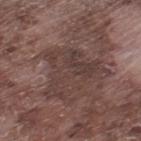biopsy_status: not biopsied; imaged during a skin examination
site: left thigh
automated_metrics:
  area_mm2_approx: 21.0
  eccentricity: 0.7
  shape_asymmetry: 0.7
  vs_skin_darker_L: 6.0
  vs_skin_contrast_norm: 5.5
  nevus_likeness_0_100: 0
image:
  source: total-body photography crop
  field_of_view_mm: 15
patient:
  sex: male
  age_approx: 75
lesion_size:
  long_diameter_mm_approx: 7.5
lighting: white-light